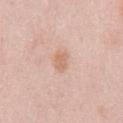Clinical impression:
This lesion was catalogued during total-body skin photography and was not selected for biopsy.
Clinical summary:
The lesion is located on the back. An algorithmic analysis of the crop reported a border-irregularity index near 2.5/10, internal color variation of about 1.5 on a 0–10 scale, and a peripheral color-asymmetry measure near 0.5. It also reported a nevus-likeness score of about 40/100 and a lesion-detection confidence of about 100/100. This image is a 15 mm lesion crop taken from a total-body photograph. A female subject, aged approximately 40. Captured under white-light illumination.